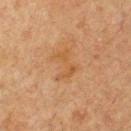Notes:
– workup · catalogued during a skin exam; not biopsied
– image · 15 mm crop, total-body photography
– subject · male, about 65 years old
– diameter · ≈3.5 mm
– body site · the chest
– lighting · cross-polarized illumination